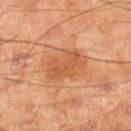<tbp_lesion>
  <biopsy_status>not biopsied; imaged during a skin examination</biopsy_status>
</tbp_lesion>